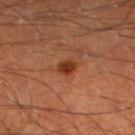biopsy status — no biopsy performed (imaged during a skin exam) | site — the left lower leg | image — 15 mm crop, total-body photography | lesion diameter — ~2.5 mm (longest diameter) | lighting — cross-polarized illumination | automated lesion analysis — an average lesion color of about L≈32 a*≈25 b*≈31 (CIELAB), roughly 10 lightness units darker than nearby skin, and a normalized border contrast of about 9; a border-irregularity index near 1.5/10, a within-lesion color-variation index near 2/10, and radial color variation of about 0.5; a classifier nevus-likeness of about 95/100 and lesion-presence confidence of about 100/100 | patient — male, roughly 65 years of age.